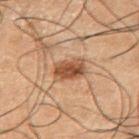Findings:
* notes: catalogued during a skin exam; not biopsied
* illumination: cross-polarized illumination
* location: the chest
* TBP lesion metrics: a footprint of about 7 mm², an outline eccentricity of about 0.7 (0 = round, 1 = elongated), and a symmetry-axis asymmetry near 0.15; a mean CIELAB color near L≈37 a*≈17 b*≈26 and about 11 CIELAB-L* units darker than the surrounding skin; a nevus-likeness score of about 90/100 and a lesion-detection confidence of about 100/100
* lesion diameter: ~3.5 mm (longest diameter)
* patient: male, about 50 years old
* imaging modality: 15 mm crop, total-body photography